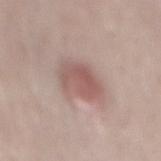Assessment:
Part of a total-body skin-imaging series; this lesion was reviewed on a skin check and was not flagged for biopsy.
Context:
Located on the lower back. Measured at roughly 4 mm in maximum diameter. A 15 mm crop from a total-body photograph taken for skin-cancer surveillance. This is a white-light tile. A male patient, aged 63–67.